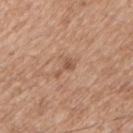Impression:
This lesion was catalogued during total-body skin photography and was not selected for biopsy.
Acquisition and patient details:
Longest diameter approximately 3 mm. From the right upper arm. A male patient, about 60 years old. Cropped from a whole-body photographic skin survey; the tile spans about 15 mm. Automated image analysis of the tile measured a lesion color around L≈54 a*≈20 b*≈30 in CIELAB, a lesion–skin lightness drop of about 9, and a lesion-to-skin contrast of about 6 (normalized; higher = more distinct). It also reported a color-variation rating of about 1/10 and radial color variation of about 0.5. It also reported a nevus-likeness score of about 0/100.